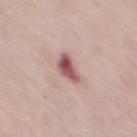| key | value |
|---|---|
| follow-up | total-body-photography surveillance lesion; no biopsy |
| lesion diameter | ≈3 mm |
| image | 15 mm crop, total-body photography |
| anatomic site | the chest |
| tile lighting | white-light illumination |
| subject | female, aged 58–62 |